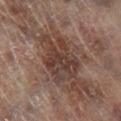The lesion was tiled from a total-body skin photograph and was not biopsied. On the right lower leg. A region of skin cropped from a whole-body photographic capture, roughly 15 mm wide. This is a cross-polarized tile. The lesion's longest dimension is about 6.5 mm. A male patient aged 63 to 67.